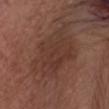follow-up: catalogued during a skin exam; not biopsied
TBP lesion metrics: a lesion area of about 25 mm², an eccentricity of roughly 0.75, and a symmetry-axis asymmetry near 0.2; an average lesion color of about L≈36 a*≈19 b*≈24 (CIELAB), a lesion–skin lightness drop of about 7, and a lesion-to-skin contrast of about 6 (normalized; higher = more distinct); an automated nevus-likeness rating near 0 out of 100 and a lesion-detection confidence of about 100/100
patient: male, aged 73 to 77
acquisition: ~15 mm tile from a whole-body skin photo
illumination: white-light illumination
body site: the head or neck
lesion size: ~7 mm (longest diameter)